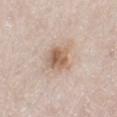notes=total-body-photography surveillance lesion; no biopsy | lesion size=about 3.5 mm | acquisition=~15 mm tile from a whole-body skin photo | tile lighting=white-light illumination | site=the leg | TBP lesion metrics=an eccentricity of roughly 0.65; about 12 CIELAB-L* units darker than the surrounding skin and a normalized border contrast of about 8; a nevus-likeness score of about 80/100 and a lesion-detection confidence of about 100/100 | patient=male, roughly 80 years of age.